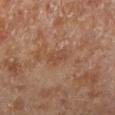Assessment: Captured during whole-body skin photography for melanoma surveillance; the lesion was not biopsied. Image and clinical context: A male patient, aged 68–72. A region of skin cropped from a whole-body photographic capture, roughly 15 mm wide. From the left lower leg.